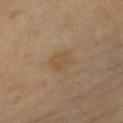| field | value |
|---|---|
| workup | no biopsy performed (imaged during a skin exam) |
| subject | female, aged around 70 |
| acquisition | ~15 mm crop, total-body skin-cancer survey |
| body site | the right forearm |